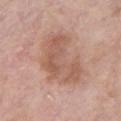No biopsy was performed on this lesion — it was imaged during a full skin examination and was not determined to be concerning. Located on the chest. A region of skin cropped from a whole-body photographic capture, roughly 15 mm wide. The patient is a female aged approximately 70. The recorded lesion diameter is about 7 mm. Imaged with white-light lighting.